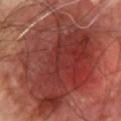follow-up — catalogued during a skin exam; not biopsied | body site — the chest | size — ~15.5 mm (longest diameter) | imaging modality — ~15 mm tile from a whole-body skin photo | subject — male, aged approximately 70 | TBP lesion metrics — an outline eccentricity of about 0.7 (0 = round, 1 = elongated) and two-axis asymmetry of about 0.2; a lesion color around L≈39 a*≈29 b*≈26 in CIELAB, a lesion–skin lightness drop of about 13, and a normalized lesion–skin contrast near 10; a nevus-likeness score of about 0/100 and lesion-presence confidence of about 100/100.